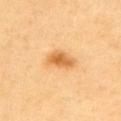<case>
  <biopsy_status>not biopsied; imaged during a skin examination</biopsy_status>
  <site>arm</site>
  <lesion_size>
    <long_diameter_mm_approx>4.0</long_diameter_mm_approx>
  </lesion_size>
  <patient>
    <sex>male</sex>
    <age_approx>60</age_approx>
  </patient>
  <image>
    <source>total-body photography crop</source>
    <field_of_view_mm>15</field_of_view_mm>
  </image>
  <lighting>cross-polarized</lighting>
  <automated_metrics>
    <area_mm2_approx>7.0</area_mm2_approx>
    <eccentricity>0.85</eccentricity>
    <shape_asymmetry>0.25</shape_asymmetry>
    <cielab_L>65</cielab_L>
    <cielab_a>24</cielab_a>
    <cielab_b>47</cielab_b>
    <vs_skin_darker_L>12.0</vs_skin_darker_L>
    <vs_skin_contrast_norm>8.0</vs_skin_contrast_norm>
  </automated_metrics>
</case>